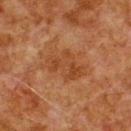Q: Is there a histopathology result?
A: no biopsy performed (imaged during a skin exam)
Q: Where on the body is the lesion?
A: the upper back
Q: Patient demographics?
A: male, roughly 80 years of age
Q: How was this image acquired?
A: 15 mm crop, total-body photography